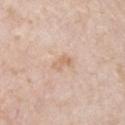Clinical impression:
Imaged during a routine full-body skin examination; the lesion was not biopsied and no histopathology is available.
Image and clinical context:
A male patient, roughly 50 years of age. The tile uses white-light illumination. A 15 mm close-up tile from a total-body photography series done for melanoma screening. The lesion is located on the chest. The lesion's longest dimension is about 2.5 mm.